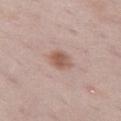Q: Was this lesion biopsied?
A: total-body-photography surveillance lesion; no biopsy
Q: How was the tile lit?
A: white-light
Q: What are the patient's age and sex?
A: female, aged approximately 30
Q: Lesion size?
A: ≈3 mm
Q: What is the anatomic site?
A: the left thigh
Q: How was this image acquired?
A: ~15 mm tile from a whole-body skin photo